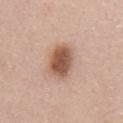Recorded during total-body skin imaging; not selected for excision or biopsy.
The patient is a male about 55 years old.
Automated image analysis of the tile measured a mean CIELAB color near L≈55 a*≈21 b*≈29 and roughly 15 lightness units darker than nearby skin.
Imaged with white-light lighting.
Cropped from a total-body skin-imaging series; the visible field is about 15 mm.